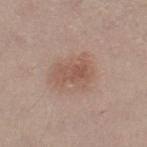Impression: Recorded during total-body skin imaging; not selected for excision or biopsy. Context: The lesion's longest dimension is about 4.5 mm. A roughly 15 mm field-of-view crop from a total-body skin photograph. The tile uses white-light illumination. The lesion is on the right thigh. A female subject, aged approximately 40.